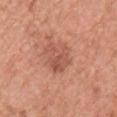notes: no biopsy performed (imaged during a skin exam); anatomic site: the back; size: ≈3.5 mm; lighting: white-light; image: 15 mm crop, total-body photography; patient: female, roughly 60 years of age.